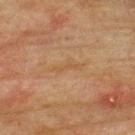Imaged during a routine full-body skin examination; the lesion was not biopsied and no histopathology is available. This is a cross-polarized tile. A 15 mm close-up extracted from a 3D total-body photography capture. The lesion's longest dimension is about 3.5 mm. Located on the upper back. The lesion-visualizer software estimated a lesion area of about 2.5 mm², a shape eccentricity near 0.95, and a symmetry-axis asymmetry near 0.65. The analysis additionally found a nevus-likeness score of about 0/100 and a lesion-detection confidence of about 95/100. The subject is a male roughly 75 years of age.